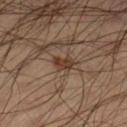Q: Was this lesion biopsied?
A: no biopsy performed (imaged during a skin exam)
Q: Patient demographics?
A: male, approximately 35 years of age
Q: Lesion location?
A: the right lower leg
Q: What kind of image is this?
A: ~15 mm tile from a whole-body skin photo